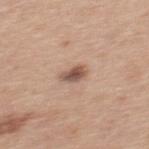Notes:
* site: the mid back
* patient: male, roughly 30 years of age
* size: about 2.5 mm
* imaging modality: total-body-photography crop, ~15 mm field of view
* TBP lesion metrics: a detector confidence of about 100 out of 100 that the crop contains a lesion